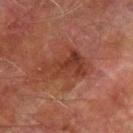biopsy status=catalogued during a skin exam; not biopsied | patient=male, aged 73–77 | body site=the arm | imaging modality=~15 mm tile from a whole-body skin photo | lighting=cross-polarized | automated metrics=a lesion area of about 13 mm², an eccentricity of roughly 0.85, and a symmetry-axis asymmetry near 0.4 | size=about 5.5 mm.